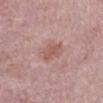biopsy status: imaged on a skin check; not biopsied.